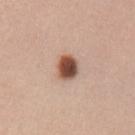Impression:
Part of a total-body skin-imaging series; this lesion was reviewed on a skin check and was not flagged for biopsy.
Acquisition and patient details:
This image is a 15 mm lesion crop taken from a total-body photograph. The recorded lesion diameter is about 3 mm. The patient is a female approximately 40 years of age. Automated tile analysis of the lesion measured an area of roughly 6 mm², an eccentricity of roughly 0.55, and a symmetry-axis asymmetry near 0.2. It also reported border irregularity of about 1.5 on a 0–10 scale and a peripheral color-asymmetry measure near 1. It also reported a classifier nevus-likeness of about 100/100 and a detector confidence of about 100 out of 100 that the crop contains a lesion. Located on the left upper arm.